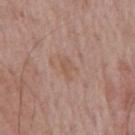Findings:
- notes · imaged on a skin check; not biopsied